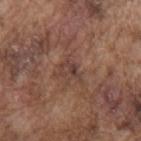Q: Illumination type?
A: white-light illumination
Q: How was this image acquired?
A: ~15 mm crop, total-body skin-cancer survey
Q: What is the lesion's diameter?
A: about 3 mm
Q: Patient demographics?
A: male, roughly 75 years of age
Q: Automated lesion metrics?
A: an outline eccentricity of about 0.75 (0 = round, 1 = elongated); a border-irregularity index near 5.5/10, internal color variation of about 1.5 on a 0–10 scale, and a peripheral color-asymmetry measure near 0.5; a classifier nevus-likeness of about 0/100
Q: Lesion location?
A: the mid back